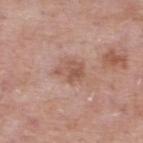| feature | finding |
|---|---|
| follow-up | catalogued during a skin exam; not biopsied |
| location | the upper back |
| size | ≈3 mm |
| image | ~15 mm crop, total-body skin-cancer survey |
| patient | male, aged 53–57 |